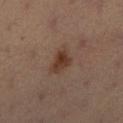Assessment:
Recorded during total-body skin imaging; not selected for excision or biopsy.
Clinical summary:
The tile uses cross-polarized illumination. On the right lower leg. The subject is a female aged 33 to 37. A 15 mm close-up tile from a total-body photography series done for melanoma screening. The lesion-visualizer software estimated an outline eccentricity of about 0.7 (0 = round, 1 = elongated) and two-axis asymmetry of about 0.3. It also reported an average lesion color of about L≈36 a*≈19 b*≈26 (CIELAB), roughly 9 lightness units darker than nearby skin, and a normalized lesion–skin contrast near 8.5.